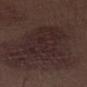<tbp_lesion>
  <biopsy_status>not biopsied; imaged during a skin examination</biopsy_status>
</tbp_lesion>